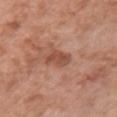Approximately 3 mm at its widest. Located on the chest. A female subject, in their 50s. This is a white-light tile. A 15 mm crop from a total-body photograph taken for skin-cancer surveillance.